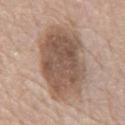Imaged during a routine full-body skin examination; the lesion was not biopsied and no histopathology is available. A male subject about 75 years old. The lesion is on the chest. A region of skin cropped from a whole-body photographic capture, roughly 15 mm wide. An algorithmic analysis of the crop reported a footprint of about 41 mm² and a shape-asymmetry score of about 0.15 (0 = symmetric). It also reported about 13 CIELAB-L* units darker than the surrounding skin and a lesion-to-skin contrast of about 9 (normalized; higher = more distinct). And it measured border irregularity of about 2.5 on a 0–10 scale and internal color variation of about 5 on a 0–10 scale.